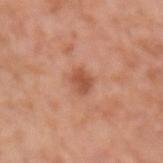– workup · total-body-photography surveillance lesion; no biopsy
– image · 15 mm crop, total-body photography
– diameter · about 2.5 mm
– lighting · white-light
– anatomic site · the left forearm
– patient · male, about 75 years old
– image-analysis metrics · an outline eccentricity of about 0.65 (0 = round, 1 = elongated) and a symmetry-axis asymmetry near 0.25; border irregularity of about 2.5 on a 0–10 scale, a within-lesion color-variation index near 2.5/10, and radial color variation of about 1